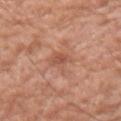Case summary:
• workup · imaged on a skin check; not biopsied
• image source · ~15 mm crop, total-body skin-cancer survey
• lesion size · about 3.5 mm
• subject · male, aged around 60
• TBP lesion metrics · a mean CIELAB color near L≈54 a*≈24 b*≈32, about 8 CIELAB-L* units darker than the surrounding skin, and a normalized lesion–skin contrast near 6; a border-irregularity rating of about 3/10, a color-variation rating of about 2.5/10, and a peripheral color-asymmetry measure near 0.5
• lighting · white-light illumination
• location · the right upper arm